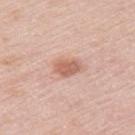Located on the left upper arm.
A 15 mm crop from a total-body photograph taken for skin-cancer surveillance.
Automated image analysis of the tile measured a mean CIELAB color near L≈61 a*≈23 b*≈29, roughly 12 lightness units darker than nearby skin, and a normalized border contrast of about 7.5. The analysis additionally found a border-irregularity rating of about 2/10.
A female patient, aged approximately 65.
Captured under white-light illumination.
Measured at roughly 3 mm in maximum diameter.